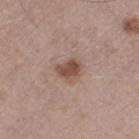Part of a total-body skin-imaging series; this lesion was reviewed on a skin check and was not flagged for biopsy. The lesion is on the right thigh. A 15 mm close-up extracted from a 3D total-body photography capture. The recorded lesion diameter is about 3 mm. A male patient, in their mid-50s. The total-body-photography lesion software estimated an outline eccentricity of about 0.6 (0 = round, 1 = elongated) and a symmetry-axis asymmetry near 0.25. It also reported an average lesion color of about L≈49 a*≈20 b*≈25 (CIELAB) and a lesion-to-skin contrast of about 8.5 (normalized; higher = more distinct). And it measured border irregularity of about 2 on a 0–10 scale and peripheral color asymmetry of about 1. This is a white-light tile.